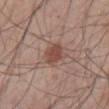Impression:
No biopsy was performed on this lesion — it was imaged during a full skin examination and was not determined to be concerning.
Image and clinical context:
The total-body-photography lesion software estimated border irregularity of about 2 on a 0–10 scale, internal color variation of about 2 on a 0–10 scale, and a peripheral color-asymmetry measure near 0.5. The software also gave a classifier nevus-likeness of about 95/100 and a detector confidence of about 100 out of 100 that the crop contains a lesion. A region of skin cropped from a whole-body photographic capture, roughly 15 mm wide. About 3 mm across. From the abdomen. A male subject aged 58 to 62.